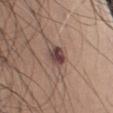{"biopsy_status": "not biopsied; imaged during a skin examination", "site": "left upper arm", "image": {"source": "total-body photography crop", "field_of_view_mm": 15}, "patient": {"sex": "male", "age_approx": 55}, "lighting": "white-light"}